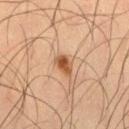Impression:
Captured during whole-body skin photography for melanoma surveillance; the lesion was not biopsied.
Background:
A 15 mm close-up tile from a total-body photography series done for melanoma screening. The patient is a male roughly 50 years of age. On the right thigh.